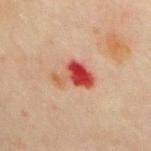{
  "biopsy_status": "not biopsied; imaged during a skin examination",
  "image": {
    "source": "total-body photography crop",
    "field_of_view_mm": 15
  },
  "lighting": "cross-polarized",
  "patient": {
    "sex": "female",
    "age_approx": 50
  },
  "site": "chest",
  "lesion_size": {
    "long_diameter_mm_approx": 4.0
  }
}